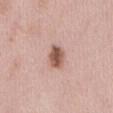This lesion was catalogued during total-body skin photography and was not selected for biopsy. A female subject about 40 years old. A lesion tile, about 15 mm wide, cut from a 3D total-body photograph. The total-body-photography lesion software estimated a lesion area of about 5 mm² and a shape-asymmetry score of about 0.15 (0 = symmetric). The analysis additionally found a lesion color around L≈54 a*≈22 b*≈27 in CIELAB. And it measured border irregularity of about 1.5 on a 0–10 scale, a within-lesion color-variation index near 3/10, and peripheral color asymmetry of about 1. The analysis additionally found an automated nevus-likeness rating near 95 out of 100. The recorded lesion diameter is about 3 mm. On the front of the torso. The tile uses white-light illumination.